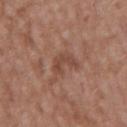Recorded during total-body skin imaging; not selected for excision or biopsy. About 3.5 mm across. The tile uses white-light illumination. The lesion is on the mid back. A male subject, aged approximately 50. This image is a 15 mm lesion crop taken from a total-body photograph.